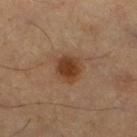Clinical impression:
No biopsy was performed on this lesion — it was imaged during a full skin examination and was not determined to be concerning.
Acquisition and patient details:
A 15 mm close-up tile from a total-body photography series done for melanoma screening. From the right lower leg. The tile uses cross-polarized illumination. A male subject, in their 60s.